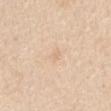subject: male, aged around 60
diameter: ~1.5 mm (longest diameter)
lighting: white-light illumination
acquisition: ~15 mm tile from a whole-body skin photo
body site: the front of the torso
TBP lesion metrics: an area of roughly 2 mm²; roughly 5 lightness units darker than nearby skin and a normalized border contrast of about 3.5; an automated nevus-likeness rating near 0 out of 100 and a lesion-detection confidence of about 100/100